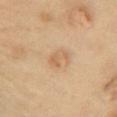{"biopsy_status": "not biopsied; imaged during a skin examination", "site": "chest", "image": {"source": "total-body photography crop", "field_of_view_mm": 15}, "patient": {"sex": "female", "age_approx": 55}}